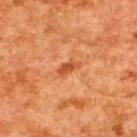biopsy status=imaged on a skin check; not biopsied | subject=male, about 60 years old | site=the upper back | image source=~15 mm crop, total-body skin-cancer survey | image-analysis metrics=a classifier nevus-likeness of about 20/100 and lesion-presence confidence of about 100/100.